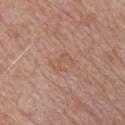Q: Is there a histopathology result?
A: imaged on a skin check; not biopsied
Q: Who is the patient?
A: male, about 70 years old
Q: What is the anatomic site?
A: the front of the torso
Q: How was this image acquired?
A: total-body-photography crop, ~15 mm field of view